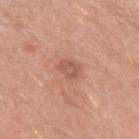Clinical impression: No biopsy was performed on this lesion — it was imaged during a full skin examination and was not determined to be concerning. Clinical summary: The lesion is located on the left upper arm. Cropped from a whole-body photographic skin survey; the tile spans about 15 mm. An algorithmic analysis of the crop reported an area of roughly 4.5 mm² and an eccentricity of roughly 0.3. The analysis additionally found border irregularity of about 2.5 on a 0–10 scale. The subject is a male aged 28–32.